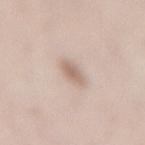notes: no biopsy performed (imaged during a skin exam)
location: the lower back
subject: female, aged around 50
lesion diameter: about 2.5 mm
image source: ~15 mm crop, total-body skin-cancer survey
automated metrics: a footprint of about 4 mm², an outline eccentricity of about 0.65 (0 = round, 1 = elongated), and two-axis asymmetry of about 0.25; a mean CIELAB color near L≈64 a*≈15 b*≈25, about 10 CIELAB-L* units darker than the surrounding skin, and a lesion-to-skin contrast of about 6.5 (normalized; higher = more distinct); a nevus-likeness score of about 95/100 and a detector confidence of about 100 out of 100 that the crop contains a lesion
tile lighting: white-light illumination